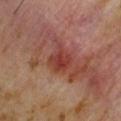Captured under cross-polarized illumination. Located on the right upper arm. Approximately 3.5 mm at its widest. Cropped from a total-body skin-imaging series; the visible field is about 15 mm. The subject is a male in their 60s.